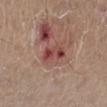Image and clinical context: Longest diameter approximately 3.5 mm. Located on the right lower leg. A male patient approximately 65 years of age. The tile uses white-light illumination. A lesion tile, about 15 mm wide, cut from a 3D total-body photograph.